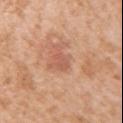  biopsy_status: not biopsied; imaged during a skin examination
  lesion_size:
    long_diameter_mm_approx: 2.5
  lighting: white-light
  automated_metrics:
    area_mm2_approx: 3.0
    eccentricity: 0.8
    shape_asymmetry: 0.3
    cielab_L: 57
    cielab_a: 25
    cielab_b: 32
    vs_skin_darker_L: 7.0
    vs_skin_contrast_norm: 5.0
    color_variation_0_10: 0.5
    peripheral_color_asymmetry: 0.0
    nevus_likeness_0_100: 0
    lesion_detection_confidence_0_100: 100
  image:
    source: total-body photography crop
    field_of_view_mm: 15
  patient:
    sex: female
    age_approx: 40
  site: right upper arm